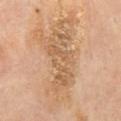Part of a total-body skin-imaging series; this lesion was reviewed on a skin check and was not flagged for biopsy.
Imaged with cross-polarized lighting.
Approximately 7.5 mm at its widest.
The patient is a female aged 58–62.
From the mid back.
The lesion-visualizer software estimated a footprint of about 15 mm², an outline eccentricity of about 0.9 (0 = round, 1 = elongated), and a shape-asymmetry score of about 0.45 (0 = symmetric). The software also gave border irregularity of about 8.5 on a 0–10 scale and peripheral color asymmetry of about 1. The analysis additionally found an automated nevus-likeness rating near 0 out of 100 and lesion-presence confidence of about 85/100.
Cropped from a total-body skin-imaging series; the visible field is about 15 mm.